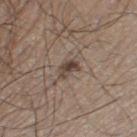This lesion was catalogued during total-body skin photography and was not selected for biopsy. A male subject aged 58–62. An algorithmic analysis of the crop reported an area of roughly 4.5 mm², an eccentricity of roughly 0.7, and a symmetry-axis asymmetry near 0.4. The analysis additionally found an automated nevus-likeness rating near 20 out of 100 and lesion-presence confidence of about 100/100. A 15 mm close-up tile from a total-body photography series done for melanoma screening. Measured at roughly 3 mm in maximum diameter. The lesion is on the left thigh. The tile uses white-light illumination.